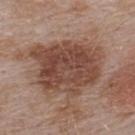Assessment: Imaged during a routine full-body skin examination; the lesion was not biopsied and no histopathology is available. Clinical summary: A male patient, aged around 55. Imaged with white-light lighting. The lesion is on the upper back. An algorithmic analysis of the crop reported an area of roughly 42 mm², a shape eccentricity near 0.6, and two-axis asymmetry of about 0.2. And it measured a lesion color around L≈46 a*≈20 b*≈26 in CIELAB and a lesion-to-skin contrast of about 9.5 (normalized; higher = more distinct). It also reported a classifier nevus-likeness of about 5/100. A 15 mm close-up extracted from a 3D total-body photography capture. About 8.5 mm across.